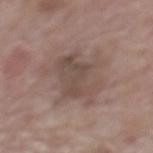Captured during whole-body skin photography for melanoma surveillance; the lesion was not biopsied.
The lesion's longest dimension is about 8 mm.
The lesion is on the upper back.
Automated image analysis of the tile measured a lesion area of about 25 mm², an eccentricity of roughly 0.8, and a symmetry-axis asymmetry near 0.35. And it measured a mean CIELAB color near L≈49 a*≈15 b*≈22 and a lesion–skin lightness drop of about 8.
A female patient, about 65 years old.
A 15 mm close-up tile from a total-body photography series done for melanoma screening.
The tile uses white-light illumination.